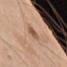biopsy status: no biopsy performed (imaged during a skin exam) | diameter: ≈3.5 mm | body site: the left upper arm | subject: male, about 65 years old | image: total-body-photography crop, ~15 mm field of view | illumination: white-light.